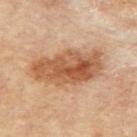Imaged during a routine full-body skin examination; the lesion was not biopsied and no histopathology is available. A roughly 15 mm field-of-view crop from a total-body skin photograph. Measured at roughly 8 mm in maximum diameter. Imaged with cross-polarized lighting. The lesion is located on the upper back. The subject is a male approximately 85 years of age or older.